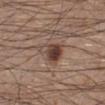This lesion was catalogued during total-body skin photography and was not selected for biopsy. This is a white-light tile. Longest diameter approximately 3.5 mm. A 15 mm close-up tile from a total-body photography series done for melanoma screening. The lesion is located on the left lower leg. A male patient, aged 28 to 32. The total-body-photography lesion software estimated two-axis asymmetry of about 0.4. The analysis additionally found a border-irregularity index near 3.5/10 and radial color variation of about 2.5. The software also gave an automated nevus-likeness rating near 95 out of 100 and a lesion-detection confidence of about 100/100.